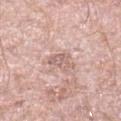Captured during whole-body skin photography for melanoma surveillance; the lesion was not biopsied. The lesion is on the left lower leg. This image is a 15 mm lesion crop taken from a total-body photograph. A male subject aged 48–52. The lesion-visualizer software estimated a footprint of about 4.5 mm² and two-axis asymmetry of about 0.55. The tile uses white-light illumination.